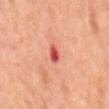notes: no biopsy performed (imaged during a skin exam)